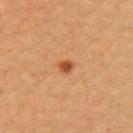notes=no biopsy performed (imaged during a skin exam)
site=the left upper arm
subject=female, roughly 35 years of age
acquisition=~15 mm crop, total-body skin-cancer survey